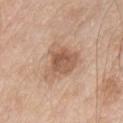tile lighting: white-light; anatomic site: the front of the torso; acquisition: ~15 mm crop, total-body skin-cancer survey; diameter: ~5 mm (longest diameter); subject: male, about 80 years old.